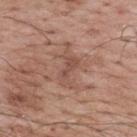follow-up: no biopsy performed (imaged during a skin exam)
image-analysis metrics: a footprint of about 8.5 mm², an outline eccentricity of about 0.9 (0 = round, 1 = elongated), and a symmetry-axis asymmetry near 0.5; an average lesion color of about L≈51 a*≈20 b*≈27 (CIELAB), about 7 CIELAB-L* units darker than the surrounding skin, and a normalized border contrast of about 5.5; an automated nevus-likeness rating near 0 out of 100 and lesion-presence confidence of about 90/100
body site: the upper back
size: about 5 mm
illumination: white-light
image source: total-body-photography crop, ~15 mm field of view
patient: male, about 70 years old